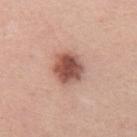Assessment: Captured during whole-body skin photography for melanoma surveillance; the lesion was not biopsied. Image and clinical context: A male subject, aged 53–57. A 15 mm close-up tile from a total-body photography series done for melanoma screening. About 4 mm across. On the front of the torso.